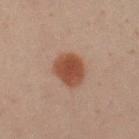Recorded during total-body skin imaging; not selected for excision or biopsy. A 15 mm close-up extracted from a 3D total-body photography capture. A male subject roughly 50 years of age. Located on the left upper arm.